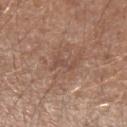  biopsy_status: not biopsied; imaged during a skin examination
  site: left forearm
  lighting: white-light
  patient:
    sex: male
    age_approx: 65
  image:
    source: total-body photography crop
    field_of_view_mm: 15
  lesion_size:
    long_diameter_mm_approx: 2.5